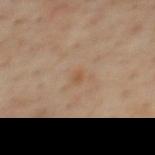Case summary:
* workup: no biopsy performed (imaged during a skin exam)
* anatomic site: the upper back
* subject: male, aged approximately 55
* acquisition: ~15 mm tile from a whole-body skin photo
* size: ≈1.5 mm